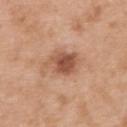| key | value |
|---|---|
| workup | imaged on a skin check; not biopsied |
| image source | total-body-photography crop, ~15 mm field of view |
| tile lighting | white-light illumination |
| subject | male, in their 50s |
| body site | the upper back |